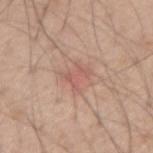<case>
<biopsy_status>not biopsied; imaged during a skin examination</biopsy_status>
<site>right forearm</site>
<image>
  <source>total-body photography crop</source>
  <field_of_view_mm>15</field_of_view_mm>
</image>
<automated_metrics>
  <eccentricity>0.65</eccentricity>
</automated_metrics>
<patient>
  <sex>male</sex>
  <age_approx>60</age_approx>
</patient>
</case>